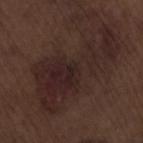Impression:
Recorded during total-body skin imaging; not selected for excision or biopsy.
Context:
A close-up tile cropped from a whole-body skin photograph, about 15 mm across. The lesion's longest dimension is about 11 mm. A male subject roughly 70 years of age. The lesion is on the lower back. The total-body-photography lesion software estimated a classifier nevus-likeness of about 0/100 and a detector confidence of about 100 out of 100 that the crop contains a lesion.